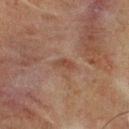workup: imaged on a skin check; not biopsied | location: the chest | image: ~15 mm tile from a whole-body skin photo | patient: male, aged 73 to 77 | automated metrics: a footprint of about 2.5 mm², an outline eccentricity of about 0.9 (0 = round, 1 = elongated), and two-axis asymmetry of about 0.4; a border-irregularity rating of about 4/10 and a peripheral color-asymmetry measure near 0; a nevus-likeness score of about 0/100 and a detector confidence of about 100 out of 100 that the crop contains a lesion | size: ~2.5 mm (longest diameter).